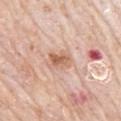{"biopsy_status": "not biopsied; imaged during a skin examination"}